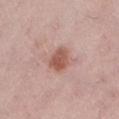Clinical impression:
The lesion was photographed on a routine skin check and not biopsied; there is no pathology result.
Context:
On the left lower leg. Automated tile analysis of the lesion measured a lesion color around L≈56 a*≈23 b*≈26 in CIELAB and a lesion-to-skin contrast of about 8 (normalized; higher = more distinct). The software also gave internal color variation of about 3 on a 0–10 scale and peripheral color asymmetry of about 1. And it measured a nevus-likeness score of about 95/100 and lesion-presence confidence of about 100/100. A lesion tile, about 15 mm wide, cut from a 3D total-body photograph. A female patient aged 48 to 52. About 3 mm across. This is a white-light tile.